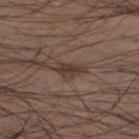On the left lower leg. A male patient approximately 40 years of age. Captured under white-light illumination. A 15 mm close-up extracted from a 3D total-body photography capture.